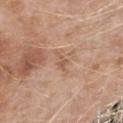The lesion was tiled from a total-body skin photograph and was not biopsied. Imaged with white-light lighting. The lesion is on the right forearm. Cropped from a whole-body photographic skin survey; the tile spans about 15 mm. The patient is a male about 75 years old. An algorithmic analysis of the crop reported a footprint of about 2.5 mm², an outline eccentricity of about 0.9 (0 = round, 1 = elongated), and two-axis asymmetry of about 0.45. And it measured roughly 8 lightness units darker than nearby skin and a lesion-to-skin contrast of about 5.5 (normalized; higher = more distinct). The software also gave a detector confidence of about 95 out of 100 that the crop contains a lesion.